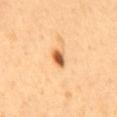Q: Was this lesion biopsied?
A: imaged on a skin check; not biopsied
Q: How was this image acquired?
A: 15 mm crop, total-body photography
Q: What lighting was used for the tile?
A: cross-polarized illumination
Q: What are the patient's age and sex?
A: female, aged 53–57
Q: Lesion location?
A: the mid back
Q: Automated lesion metrics?
A: a border-irregularity rating of about 2/10, a within-lesion color-variation index near 4.5/10, and radial color variation of about 1; a nevus-likeness score of about 95/100 and a detector confidence of about 100 out of 100 that the crop contains a lesion
Q: Lesion size?
A: ≈2.5 mm